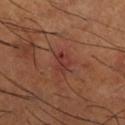Context:
A region of skin cropped from a whole-body photographic capture, roughly 15 mm wide. From the right lower leg. Measured at roughly 2.5 mm in maximum diameter. An algorithmic analysis of the crop reported an average lesion color of about L≈36 a*≈27 b*≈26 (CIELAB) and about 7 CIELAB-L* units darker than the surrounding skin. It also reported a border-irregularity rating of about 2.5/10, a within-lesion color-variation index near 2.5/10, and radial color variation of about 1. The analysis additionally found a classifier nevus-likeness of about 0/100. The subject is a male approximately 65 years of age.